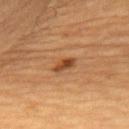{
  "biopsy_status": "not biopsied; imaged during a skin examination",
  "patient": {
    "sex": "male",
    "age_approx": "85+"
  },
  "image": {
    "source": "total-body photography crop",
    "field_of_view_mm": 15
  },
  "site": "upper back"
}